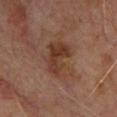Recorded during total-body skin imaging; not selected for excision or biopsy.